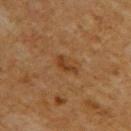Part of a total-body skin-imaging series; this lesion was reviewed on a skin check and was not flagged for biopsy.